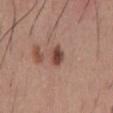Findings:
* biopsy status — no biopsy performed (imaged during a skin exam)